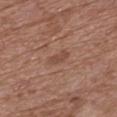This lesion was catalogued during total-body skin photography and was not selected for biopsy.
The patient is a female roughly 75 years of age.
The lesion is on the chest.
Cropped from a whole-body photographic skin survey; the tile spans about 15 mm.
Approximately 3 mm at its widest.